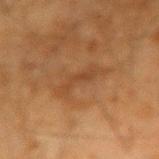Imaged during a routine full-body skin examination; the lesion was not biopsied and no histopathology is available.
This image is a 15 mm lesion crop taken from a total-body photograph.
The subject is a male roughly 65 years of age.
Located on the right upper arm.